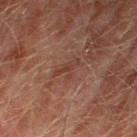| feature | finding |
|---|---|
| biopsy status | imaged on a skin check; not biopsied |
| tile lighting | cross-polarized illumination |
| lesion size | ≈3.5 mm |
| automated metrics | a border-irregularity rating of about 8/10, a within-lesion color-variation index near 0/10, and peripheral color asymmetry of about 0 |
| patient | male, aged approximately 75 |
| location | the leg |
| image source | total-body-photography crop, ~15 mm field of view |